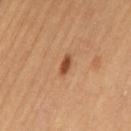Q: Is there a histopathology result?
A: total-body-photography surveillance lesion; no biopsy
Q: What is the lesion's diameter?
A: ~2.5 mm (longest diameter)
Q: Lesion location?
A: the leg
Q: How was the tile lit?
A: cross-polarized
Q: How was this image acquired?
A: total-body-photography crop, ~15 mm field of view
Q: Patient demographics?
A: female, about 65 years old